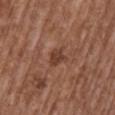Case summary:
* follow-up — catalogued during a skin exam; not biopsied
* anatomic site — the mid back
* imaging modality — 15 mm crop, total-body photography
* diameter — about 2.5 mm
* subject — male, roughly 75 years of age
* illumination — white-light illumination
* TBP lesion metrics — a lesion color around L≈40 a*≈22 b*≈27 in CIELAB, a lesion–skin lightness drop of about 9, and a lesion-to-skin contrast of about 7.5 (normalized; higher = more distinct)